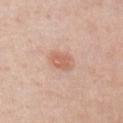Clinical impression:
Imaged during a routine full-body skin examination; the lesion was not biopsied and no histopathology is available.
Image and clinical context:
A roughly 15 mm field-of-view crop from a total-body skin photograph. A male patient, approximately 60 years of age. From the arm. Imaged with white-light lighting. Approximately 4 mm at its widest.